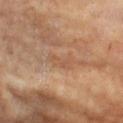Clinical impression:
Imaged during a routine full-body skin examination; the lesion was not biopsied and no histopathology is available.
Acquisition and patient details:
The tile uses cross-polarized illumination. A female subject, aged around 65. Approximately 3.5 mm at its widest. The total-body-photography lesion software estimated a footprint of about 5 mm² and a shape-asymmetry score of about 0.4 (0 = symmetric). The analysis additionally found about 6 CIELAB-L* units darker than the surrounding skin and a lesion-to-skin contrast of about 4.5 (normalized; higher = more distinct). Located on the chest. A 15 mm close-up tile from a total-body photography series done for melanoma screening.